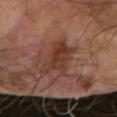notes = no biopsy performed (imaged during a skin exam); tile lighting = cross-polarized illumination; image source = total-body-photography crop, ~15 mm field of view; subject = male, aged around 65; size = about 5.5 mm; body site = the right forearm.